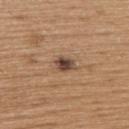Clinical impression:
The lesion was photographed on a routine skin check and not biopsied; there is no pathology result.
Context:
The lesion is on the back. This is a white-light tile. A roughly 15 mm field-of-view crop from a total-body skin photograph. The recorded lesion diameter is about 2.5 mm. The patient is a male about 65 years old.